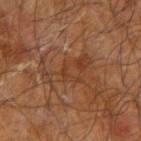Imaged during a routine full-body skin examination; the lesion was not biopsied and no histopathology is available.
A male subject, approximately 70 years of age.
A close-up tile cropped from a whole-body skin photograph, about 15 mm across.
Approximately 5.5 mm at its widest.
The lesion is on the left forearm.